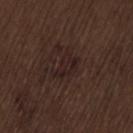{"biopsy_status": "not biopsied; imaged during a skin examination", "automated_metrics": {"cielab_L": 20, "cielab_a": 15, "cielab_b": 16, "vs_skin_darker_L": 5.0, "vs_skin_contrast_norm": 6.5, "border_irregularity_0_10": 3.5, "color_variation_0_10": 2.5, "peripheral_color_asymmetry": 0.5, "nevus_likeness_0_100": 10}, "patient": {"sex": "male", "age_approx": 70}, "lighting": "white-light", "lesion_size": {"long_diameter_mm_approx": 3.0}, "site": "lower back", "image": {"source": "total-body photography crop", "field_of_view_mm": 15}}